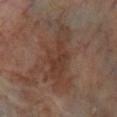Impression:
No biopsy was performed on this lesion — it was imaged during a full skin examination and was not determined to be concerning.
Context:
A 15 mm close-up extracted from a 3D total-body photography capture. About 8.5 mm across. The tile uses cross-polarized illumination. A male subject, roughly 70 years of age. On the right lower leg.